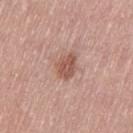Q: Is there a histopathology result?
A: no biopsy performed (imaged during a skin exam)
Q: How large is the lesion?
A: ≈3 mm
Q: Patient demographics?
A: female, aged 63–67
Q: What is the imaging modality?
A: ~15 mm tile from a whole-body skin photo
Q: What did automated image analysis measure?
A: border irregularity of about 2.5 on a 0–10 scale, internal color variation of about 2 on a 0–10 scale, and a peripheral color-asymmetry measure near 1
Q: Where on the body is the lesion?
A: the left thigh